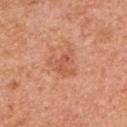Impression: The lesion was photographed on a routine skin check and not biopsied; there is no pathology result.